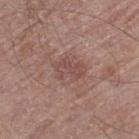Acquisition and patient details: Captured under white-light illumination. A male patient in their 70s. A lesion tile, about 15 mm wide, cut from a 3D total-body photograph. The lesion is located on the left thigh.